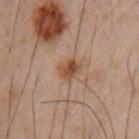<tbp_lesion>
  <biopsy_status>not biopsied; imaged during a skin examination</biopsy_status>
  <image>
    <source>total-body photography crop</source>
    <field_of_view_mm>15</field_of_view_mm>
  </image>
  <lesion_size>
    <long_diameter_mm_approx>3.0</long_diameter_mm_approx>
  </lesion_size>
  <site>left upper arm</site>
  <lighting>cross-polarized</lighting>
  <patient>
    <sex>male</sex>
    <age_approx>50</age_approx>
  </patient>
</tbp_lesion>